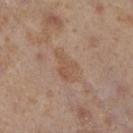Captured during whole-body skin photography for melanoma surveillance; the lesion was not biopsied.
About 4 mm across.
The total-body-photography lesion software estimated a shape eccentricity near 0.85 and two-axis asymmetry of about 0.35. It also reported a color-variation rating of about 2.5/10 and peripheral color asymmetry of about 0.5.
From the right lower leg.
The tile uses cross-polarized illumination.
The patient is a female in their 40s.
This image is a 15 mm lesion crop taken from a total-body photograph.